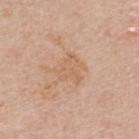biopsy status — catalogued during a skin exam; not biopsied | size — about 4.5 mm | image-analysis metrics — roughly 6 lightness units darker than nearby skin; border irregularity of about 6.5 on a 0–10 scale and a within-lesion color-variation index near 2/10 | imaging modality — 15 mm crop, total-body photography | anatomic site — the upper back | tile lighting — white-light | subject — male, aged around 65.